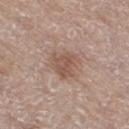| field | value |
|---|---|
| location | the right thigh |
| subject | female, in their mid- to late 70s |
| tile lighting | white-light |
| imaging modality | ~15 mm tile from a whole-body skin photo |
| TBP lesion metrics | a within-lesion color-variation index near 2.5/10 and radial color variation of about 1; a lesion-detection confidence of about 100/100 |